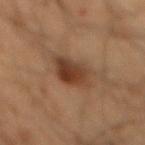biopsy_status: not biopsied; imaged during a skin examination
lighting: cross-polarized
lesion_size:
  long_diameter_mm_approx: 4.0
patient:
  sex: male
  age_approx: 50
image:
  source: total-body photography crop
  field_of_view_mm: 15
site: mid back